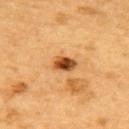Findings:
* workup · catalogued during a skin exam; not biopsied
* size · ≈3 mm
* anatomic site · the upper back
* imaging modality · ~15 mm tile from a whole-body skin photo
* patient · male, about 85 years old or older